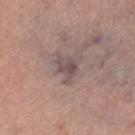Assessment: The lesion was photographed on a routine skin check and not biopsied; there is no pathology result. Clinical summary: A 15 mm close-up extracted from a 3D total-body photography capture. The lesion is on the right lower leg. The subject is a female in their 60s.